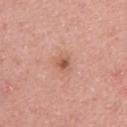Recorded during total-body skin imaging; not selected for excision or biopsy. About 1.5 mm across. Cropped from a total-body skin-imaging series; the visible field is about 15 mm. On the right upper arm. Imaged with white-light lighting. The subject is a female aged around 40.